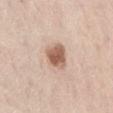  biopsy_status: not biopsied; imaged during a skin examination
  image:
    source: total-body photography crop
    field_of_view_mm: 15
  patient:
    sex: male
    age_approx: 75
  site: abdomen
  lighting: white-light